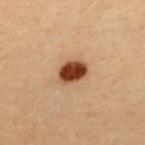Assessment: The lesion was photographed on a routine skin check and not biopsied; there is no pathology result. Clinical summary: Captured under cross-polarized illumination. A female subject, aged around 30. The lesion is on the upper back. The recorded lesion diameter is about 3.5 mm. Cropped from a total-body skin-imaging series; the visible field is about 15 mm.